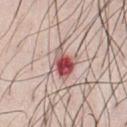Findings:
• biopsy status — catalogued during a skin exam; not biopsied